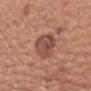follow-up: imaged on a skin check; not biopsied
TBP lesion metrics: an average lesion color of about L≈49 a*≈24 b*≈26 (CIELAB) and a lesion–skin lightness drop of about 10
acquisition: total-body-photography crop, ~15 mm field of view
patient: female, aged 58 to 62
anatomic site: the left upper arm
illumination: white-light illumination